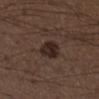The lesion was tiled from a total-body skin photograph and was not biopsied.